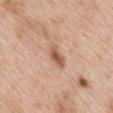Recorded during total-body skin imaging; not selected for excision or biopsy. The lesion is on the mid back. An algorithmic analysis of the crop reported a footprint of about 4.5 mm² and a symmetry-axis asymmetry near 0.25. The software also gave a lesion color around L≈57 a*≈21 b*≈31 in CIELAB and a lesion-to-skin contrast of about 8 (normalized; higher = more distinct). And it measured a classifier nevus-likeness of about 80/100 and a detector confidence of about 100 out of 100 that the crop contains a lesion. A female subject, aged approximately 40. A roughly 15 mm field-of-view crop from a total-body skin photograph.